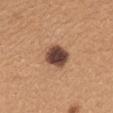– notes — no biopsy performed (imaged during a skin exam)
– patient — female, roughly 40 years of age
– tile lighting — white-light illumination
– image — ~15 mm tile from a whole-body skin photo
– lesion diameter — ≈3.5 mm
– automated lesion analysis — an area of roughly 8 mm², an eccentricity of roughly 0.55, and two-axis asymmetry of about 0.15
– site — the mid back